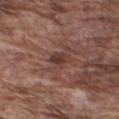notes = catalogued during a skin exam; not biopsied | lesion size = about 2.5 mm | imaging modality = ~15 mm crop, total-body skin-cancer survey | illumination = white-light | subject = male, aged approximately 75 | automated lesion analysis = a border-irregularity index near 3/10 and internal color variation of about 3.5 on a 0–10 scale; an automated nevus-likeness rating near 0 out of 100 and lesion-presence confidence of about 95/100 | anatomic site = the right upper arm.